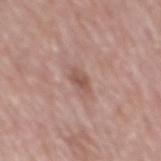follow-up: no biopsy performed (imaged during a skin exam)
lesion size: ≈2.5 mm
acquisition: ~15 mm crop, total-body skin-cancer survey
automated lesion analysis: a footprint of about 3 mm², an outline eccentricity of about 0.85 (0 = round, 1 = elongated), and a symmetry-axis asymmetry near 0.25; an average lesion color of about L≈53 a*≈20 b*≈24 (CIELAB), about 10 CIELAB-L* units darker than the surrounding skin, and a normalized lesion–skin contrast near 7
subject: male, in their 70s
illumination: white-light illumination
location: the mid back